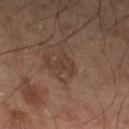No biopsy was performed on this lesion — it was imaged during a full skin examination and was not determined to be concerning. A 15 mm crop from a total-body photograph taken for skin-cancer surveillance. Located on the left lower leg. The total-body-photography lesion software estimated a lesion area of about 4 mm², an outline eccentricity of about 0.6 (0 = round, 1 = elongated), and two-axis asymmetry of about 0.5. This is a cross-polarized tile. The recorded lesion diameter is about 2.5 mm. A male subject about 55 years old.